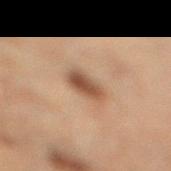notes: imaged on a skin check; not biopsied
site: the leg
acquisition: total-body-photography crop, ~15 mm field of view
patient: male, aged around 70